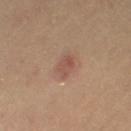Captured during whole-body skin photography for melanoma surveillance; the lesion was not biopsied. Imaged with cross-polarized lighting. Longest diameter approximately 3 mm. The lesion is located on the right thigh. A 15 mm close-up extracted from a 3D total-body photography capture. The subject is a female approximately 55 years of age. Automated image analysis of the tile measured an area of roughly 4 mm², an eccentricity of roughly 0.8, and a shape-asymmetry score of about 0.25 (0 = symmetric). And it measured about 8 CIELAB-L* units darker than the surrounding skin and a normalized lesion–skin contrast near 6. And it measured a classifier nevus-likeness of about 60/100 and lesion-presence confidence of about 100/100.